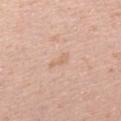Clinical impression:
The lesion was tiled from a total-body skin photograph and was not biopsied.
Acquisition and patient details:
Automated tile analysis of the lesion measured a footprint of about 2.5 mm², an outline eccentricity of about 0.9 (0 = round, 1 = elongated), and two-axis asymmetry of about 0.35. The analysis additionally found a mean CIELAB color near L≈67 a*≈19 b*≈31 and a lesion-to-skin contrast of about 5 (normalized; higher = more distinct). The analysis additionally found an automated nevus-likeness rating near 0 out of 100. Located on the left forearm. The tile uses white-light illumination. A female patient, about 30 years old. This image is a 15 mm lesion crop taken from a total-body photograph. About 2.5 mm across.